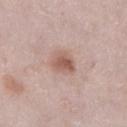  biopsy_status: not biopsied; imaged during a skin examination
  patient:
    sex: female
    age_approx: 30
  image:
    source: total-body photography crop
    field_of_view_mm: 15
  lighting: white-light
  lesion_size:
    long_diameter_mm_approx: 3.0
  site: left lower leg
  automated_metrics:
    cielab_L: 57
    cielab_a: 20
    cielab_b: 25